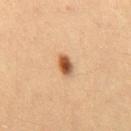anatomic site = the right thigh
TBP lesion metrics = a footprint of about 4 mm², an eccentricity of roughly 0.8, and two-axis asymmetry of about 0.2; a within-lesion color-variation index near 7.5/10 and peripheral color asymmetry of about 2.5
illumination = cross-polarized illumination
subject = female, in their 30s
image source = total-body-photography crop, ~15 mm field of view
lesion diameter = about 3 mm